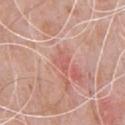Case summary:
* follow-up: total-body-photography surveillance lesion; no biopsy
* patient: male, in their 50s
* tile lighting: white-light
* image source: ~15 mm tile from a whole-body skin photo
* automated metrics: a mean CIELAB color near L≈59 a*≈28 b*≈27, about 7 CIELAB-L* units darker than the surrounding skin, and a normalized border contrast of about 5; a border-irregularity index near 3.5/10, internal color variation of about 1.5 on a 0–10 scale, and peripheral color asymmetry of about 0.5; lesion-presence confidence of about 100/100
* anatomic site: the chest